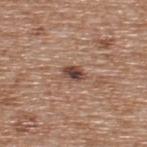This lesion was catalogued during total-body skin photography and was not selected for biopsy. A male subject, aged 63 to 67. Captured under white-light illumination. On the upper back. Longest diameter approximately 2.5 mm. A 15 mm close-up tile from a total-body photography series done for melanoma screening. The lesion-visualizer software estimated an area of roughly 4 mm² and a symmetry-axis asymmetry near 0.25. The analysis additionally found an average lesion color of about L≈45 a*≈19 b*≈25 (CIELAB). The analysis additionally found a border-irregularity index near 2.5/10 and a within-lesion color-variation index near 5/10. The analysis additionally found an automated nevus-likeness rating near 70 out of 100 and lesion-presence confidence of about 100/100.